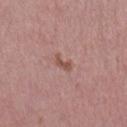Q: Was this lesion biopsied?
A: no biopsy performed (imaged during a skin exam)
Q: Where on the body is the lesion?
A: the right lower leg
Q: What did automated image analysis measure?
A: a lesion area of about 3 mm²
Q: How was this image acquired?
A: 15 mm crop, total-body photography
Q: What are the patient's age and sex?
A: female, aged approximately 40